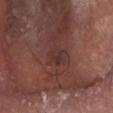Impression: This lesion was catalogued during total-body skin photography and was not selected for biopsy. Context: The patient is a male aged 78 to 82. Approximately 2.5 mm at its widest. A 15 mm close-up extracted from a 3D total-body photography capture. Captured under white-light illumination. The lesion is located on the head or neck.